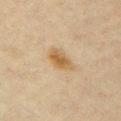Clinical impression:
Recorded during total-body skin imaging; not selected for excision or biopsy.
Acquisition and patient details:
Approximately 4 mm at its widest. Located on the front of the torso. The tile uses cross-polarized illumination. A female subject aged 43–47. Automated tile analysis of the lesion measured a lesion area of about 6.5 mm², an eccentricity of roughly 0.85, and a symmetry-axis asymmetry near 0.2. It also reported a classifier nevus-likeness of about 70/100 and a lesion-detection confidence of about 100/100. A 15 mm close-up extracted from a 3D total-body photography capture.